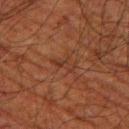Q: Is there a histopathology result?
A: catalogued during a skin exam; not biopsied
Q: Lesion location?
A: the left thigh
Q: Who is the patient?
A: male, aged approximately 80
Q: What is the imaging modality?
A: total-body-photography crop, ~15 mm field of view